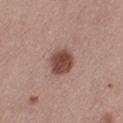Imaged during a routine full-body skin examination; the lesion was not biopsied and no histopathology is available. An algorithmic analysis of the crop reported a lesion color around L≈46 a*≈21 b*≈24 in CIELAB, about 14 CIELAB-L* units darker than the surrounding skin, and a normalized border contrast of about 10.5. It also reported an automated nevus-likeness rating near 100 out of 100 and a lesion-detection confidence of about 100/100. The tile uses white-light illumination. A 15 mm close-up extracted from a 3D total-body photography capture. A female patient about 55 years old. Longest diameter approximately 3.5 mm. Located on the leg.